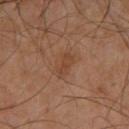{
  "biopsy_status": "not biopsied; imaged during a skin examination",
  "patient": {
    "sex": "male",
    "age_approx": 60
  },
  "lesion_size": {
    "long_diameter_mm_approx": 3.5
  },
  "site": "chest",
  "image": {
    "source": "total-body photography crop",
    "field_of_view_mm": 15
  },
  "automated_metrics": {
    "cielab_L": 39,
    "cielab_a": 18,
    "cielab_b": 28,
    "vs_skin_darker_L": 5.0,
    "vs_skin_contrast_norm": 5.5,
    "color_variation_0_10": 1.5,
    "peripheral_color_asymmetry": 0.5,
    "nevus_likeness_0_100": 0,
    "lesion_detection_confidence_0_100": 100
  },
  "lighting": "cross-polarized"
}